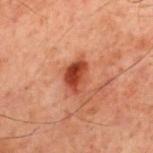| key | value |
|---|---|
| illumination | cross-polarized illumination |
| size | about 3.5 mm |
| patient | male, aged around 60 |
| acquisition | ~15 mm tile from a whole-body skin photo |
| automated metrics | a footprint of about 7.5 mm² and a shape-asymmetry score of about 0.3 (0 = symmetric); an average lesion color of about L≈35 a*≈26 b*≈29 (CIELAB), a lesion–skin lightness drop of about 12, and a normalized border contrast of about 10; a within-lesion color-variation index near 6/10 and a peripheral color-asymmetry measure near 2.5 |
| body site | the mid back |